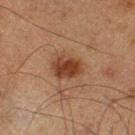{"biopsy_status": "not biopsied; imaged during a skin examination", "lighting": "cross-polarized", "patient": {"sex": "male", "age_approx": 75}, "image": {"source": "total-body photography crop", "field_of_view_mm": 15}, "site": "leg", "lesion_size": {"long_diameter_mm_approx": 3.5}, "automated_metrics": {"cielab_L": 31, "cielab_a": 20, "cielab_b": 27, "vs_skin_contrast_norm": 10.0, "nevus_likeness_0_100": 100, "lesion_detection_confidence_0_100": 100}}